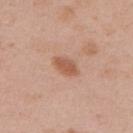The lesion was photographed on a routine skin check and not biopsied; there is no pathology result. A close-up tile cropped from a whole-body skin photograph, about 15 mm across. A female patient in their 50s. Measured at roughly 3.5 mm in maximum diameter. Imaged with white-light lighting. The lesion is located on the back.